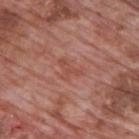No biopsy was performed on this lesion — it was imaged during a full skin examination and was not determined to be concerning. An algorithmic analysis of the crop reported an average lesion color of about L≈50 a*≈26 b*≈28 (CIELAB), roughly 6 lightness units darker than nearby skin, and a normalized border contrast of about 4.5. It also reported a lesion-detection confidence of about 100/100. The tile uses white-light illumination. Cropped from a whole-body photographic skin survey; the tile spans about 15 mm. The patient is a male aged 68–72. Measured at roughly 3.5 mm in maximum diameter. The lesion is on the upper back.